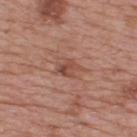workup — imaged on a skin check; not biopsied | location — the upper back | patient — male, aged around 75 | TBP lesion metrics — a lesion area of about 5 mm², an eccentricity of roughly 0.75, and a shape-asymmetry score of about 0.4 (0 = symmetric); a classifier nevus-likeness of about 0/100 and lesion-presence confidence of about 100/100 | size — about 3.5 mm | tile lighting — white-light | image source — ~15 mm crop, total-body skin-cancer survey.